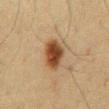{"image": {"source": "total-body photography crop", "field_of_view_mm": 15}, "automated_metrics": {"cielab_L": 36, "cielab_a": 16, "cielab_b": 28, "vs_skin_contrast_norm": 11.0, "peripheral_color_asymmetry": 2.0, "nevus_likeness_0_100": 100, "lesion_detection_confidence_0_100": 100}, "patient": {"sex": "male", "age_approx": 60}, "lighting": "cross-polarized", "site": "front of the torso"}